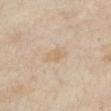Notes:
* biopsy status: no biopsy performed (imaged during a skin exam)
* lighting: cross-polarized illumination
* image source: ~15 mm tile from a whole-body skin photo
* patient: female, aged approximately 35
* body site: the chest
* TBP lesion metrics: an area of roughly 4.5 mm², an eccentricity of roughly 0.7, and a symmetry-axis asymmetry near 0.3; a lesion color around L≈63 a*≈13 b*≈31 in CIELAB, about 5 CIELAB-L* units darker than the surrounding skin, and a normalized lesion–skin contrast near 5; a border-irregularity index near 3/10, a within-lesion color-variation index near 1.5/10, and radial color variation of about 0.5; a nevus-likeness score of about 0/100 and a detector confidence of about 100 out of 100 that the crop contains a lesion